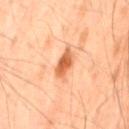A male patient aged 48 to 52.
A close-up tile cropped from a whole-body skin photograph, about 15 mm across.
The recorded lesion diameter is about 3 mm.
Located on the mid back.
Captured under cross-polarized illumination.
Automated tile analysis of the lesion measured a footprint of about 5 mm², an eccentricity of roughly 0.85, and two-axis asymmetry of about 0.2. The analysis additionally found a border-irregularity index near 2/10, a color-variation rating of about 3/10, and radial color variation of about 1.